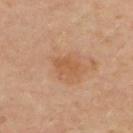No biopsy was performed on this lesion — it was imaged during a full skin examination and was not determined to be concerning.
A female subject aged approximately 50.
Located on the chest.
Automated image analysis of the tile measured a lesion area of about 5 mm² and a shape eccentricity near 0.8. And it measured a mean CIELAB color near L≈53 a*≈22 b*≈35, roughly 7 lightness units darker than nearby skin, and a normalized border contrast of about 6. The analysis additionally found a border-irregularity rating of about 2.5/10, internal color variation of about 2 on a 0–10 scale, and a peripheral color-asymmetry measure near 0.5.
A roughly 15 mm field-of-view crop from a total-body skin photograph.
The tile uses cross-polarized illumination.
Longest diameter approximately 3 mm.